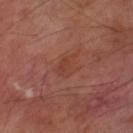No biopsy was performed on this lesion — it was imaged during a full skin examination and was not determined to be concerning.